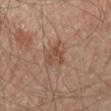Assessment:
Recorded during total-body skin imaging; not selected for excision or biopsy.
Background:
A region of skin cropped from a whole-body photographic capture, roughly 15 mm wide. About 2.5 mm across. From the leg. The subject is a male aged around 65.